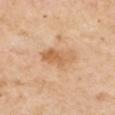biopsy status: catalogued during a skin exam; not biopsied
TBP lesion metrics: a lesion color around L≈62 a*≈20 b*≈38 in CIELAB, roughly 9 lightness units darker than nearby skin, and a normalized lesion–skin contrast near 6.5; border irregularity of about 3 on a 0–10 scale, internal color variation of about 5 on a 0–10 scale, and peripheral color asymmetry of about 1.5; a classifier nevus-likeness of about 5/100 and a lesion-detection confidence of about 100/100
patient: male, aged 53 to 57
anatomic site: the arm
diameter: ~4 mm (longest diameter)
tile lighting: cross-polarized illumination
acquisition: 15 mm crop, total-body photography